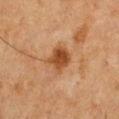Clinical summary: The lesion is located on the chest. This is a cross-polarized tile. The lesion-visualizer software estimated a footprint of about 6.5 mm², an outline eccentricity of about 0.6 (0 = round, 1 = elongated), and a symmetry-axis asymmetry near 0.25. The software also gave a border-irregularity rating of about 2.5/10, internal color variation of about 3.5 on a 0–10 scale, and a peripheral color-asymmetry measure near 1. The software also gave lesion-presence confidence of about 100/100. A male subject, in their 50s. The recorded lesion diameter is about 3.5 mm. A roughly 15 mm field-of-view crop from a total-body skin photograph.